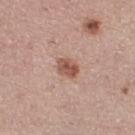Assessment:
The lesion was tiled from a total-body skin photograph and was not biopsied.